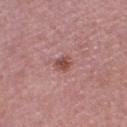<tbp_lesion>
<biopsy_status>not biopsied; imaged during a skin examination</biopsy_status>
<automated_metrics>
  <area_mm2_approx>4.0</area_mm2_approx>
  <cielab_L>50</cielab_L>
  <cielab_a>25</cielab_a>
  <cielab_b>24</cielab_b>
  <vs_skin_darker_L>10.0</vs_skin_darker_L>
  <vs_skin_contrast_norm>7.5</vs_skin_contrast_norm>
  <border_irregularity_0_10>2.5</border_irregularity_0_10>
  <nevus_likeness_0_100>80</nevus_likeness_0_100>
  <lesion_detection_confidence_0_100>100</lesion_detection_confidence_0_100>
</automated_metrics>
<image>
  <source>total-body photography crop</source>
  <field_of_view_mm>15</field_of_view_mm>
</image>
<lesion_size>
  <long_diameter_mm_approx>2.5</long_diameter_mm_approx>
</lesion_size>
<patient>
  <sex>female</sex>
  <age_approx>50</age_approx>
</patient>
<lighting>white-light</lighting>
<site>left lower leg</site>
</tbp_lesion>